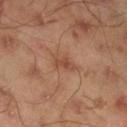Q: Patient demographics?
A: male, approximately 45 years of age
Q: Lesion location?
A: the right lower leg
Q: What kind of image is this?
A: total-body-photography crop, ~15 mm field of view
Q: What is the lesion's diameter?
A: ~2.5 mm (longest diameter)